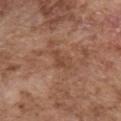{
  "lighting": "white-light",
  "image": {
    "source": "total-body photography crop",
    "field_of_view_mm": 15
  },
  "patient": {
    "sex": "male",
    "age_approx": 75
  },
  "site": "chest",
  "lesion_size": {
    "long_diameter_mm_approx": 3.0
  },
  "automated_metrics": {
    "cielab_L": 45,
    "cielab_a": 21,
    "cielab_b": 31,
    "vs_skin_darker_L": 7.0,
    "vs_skin_contrast_norm": 5.5,
    "border_irregularity_0_10": 5.5,
    "color_variation_0_10": 0.5
  }
}